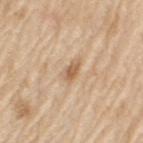Recorded during total-body skin imaging; not selected for excision or biopsy. A male subject, roughly 70 years of age. A region of skin cropped from a whole-body photographic capture, roughly 15 mm wide. About 3 mm across. From the left upper arm.